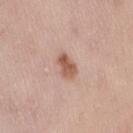biopsy status=no biopsy performed (imaged during a skin exam)
lesion size=≈3 mm
anatomic site=the right thigh
patient=female, aged 38 to 42
lighting=white-light illumination
image=15 mm crop, total-body photography